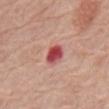This lesion was catalogued during total-body skin photography and was not selected for biopsy. Imaged with white-light lighting. From the abdomen. The patient is a male aged around 80. Cropped from a total-body skin-imaging series; the visible field is about 15 mm.